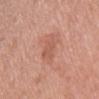biopsy_status: not biopsied; imaged during a skin examination
patient:
  sex: female
  age_approx: 40
site: front of the torso
lesion_size:
  long_diameter_mm_approx: 3.0
image:
  source: total-body photography crop
  field_of_view_mm: 15
lighting: white-light